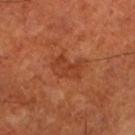Case summary:
- patient · male, aged 68–72
- lighting · cross-polarized illumination
- image · 15 mm crop, total-body photography
- anatomic site · the left lower leg
- image-analysis metrics · border irregularity of about 3.5 on a 0–10 scale and internal color variation of about 2 on a 0–10 scale; lesion-presence confidence of about 100/100